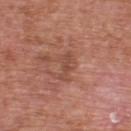workup: catalogued during a skin exam; not biopsied | automated metrics: a mean CIELAB color near L≈48 a*≈24 b*≈28, about 7 CIELAB-L* units darker than the surrounding skin, and a normalized border contrast of about 5.5; a border-irregularity index near 6/10, internal color variation of about 0 on a 0–10 scale, and radial color variation of about 0 | illumination: white-light illumination | patient: male, aged around 70 | body site: the upper back | imaging modality: total-body-photography crop, ~15 mm field of view | size: ~3 mm (longest diameter).